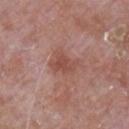Case summary:
– follow-up · no biopsy performed (imaged during a skin exam)
– subject · male, about 65 years old
– diameter · ~4 mm (longest diameter)
– tile lighting · white-light
– image source · ~15 mm crop, total-body skin-cancer survey
– body site · the upper back
– automated lesion analysis · an area of roughly 6 mm², an eccentricity of roughly 0.75, and two-axis asymmetry of about 0.35